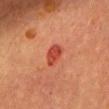Imaged during a routine full-body skin examination; the lesion was not biopsied and no histopathology is available.